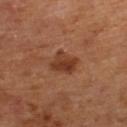Notes:
- follow-up: catalogued during a skin exam; not biopsied
- image source: ~15 mm crop, total-body skin-cancer survey
- patient: female, in their mid- to late 60s
- body site: the right lower leg